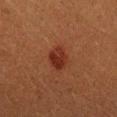The lesion was tiled from a total-body skin photograph and was not biopsied.
A 15 mm close-up extracted from a 3D total-body photography capture.
The patient is a male approximately 40 years of age.
The lesion-visualizer software estimated a lesion area of about 6 mm² and two-axis asymmetry of about 0.2. And it measured an average lesion color of about L≈26 a*≈24 b*≈26 (CIELAB). The analysis additionally found border irregularity of about 2 on a 0–10 scale, a color-variation rating of about 3.5/10, and radial color variation of about 1.5.
The lesion is on the arm.
About 3.5 mm across.
The tile uses cross-polarized illumination.